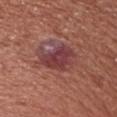| feature | finding |
|---|---|
| follow-up | no biopsy performed (imaged during a skin exam) |
| patient | male, aged 63–67 |
| lesion diameter | ~5 mm (longest diameter) |
| image | ~15 mm crop, total-body skin-cancer survey |
| anatomic site | the head or neck |
| image-analysis metrics | a lesion area of about 14 mm² and a symmetry-axis asymmetry near 0.35; an average lesion color of about L≈41 a*≈26 b*≈20 (CIELAB), about 9 CIELAB-L* units darker than the surrounding skin, and a normalized lesion–skin contrast near 8.5; an automated nevus-likeness rating near 40 out of 100 and lesion-presence confidence of about 100/100 |